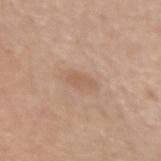{
  "biopsy_status": "not biopsied; imaged during a skin examination",
  "image": {
    "source": "total-body photography crop",
    "field_of_view_mm": 15
  },
  "lighting": "white-light",
  "site": "left forearm",
  "patient": {
    "sex": "female",
    "age_approx": 70
  },
  "lesion_size": {
    "long_diameter_mm_approx": 2.5
  }
}